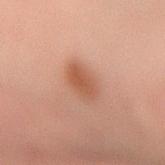Part of a total-body skin-imaging series; this lesion was reviewed on a skin check and was not flagged for biopsy. A close-up tile cropped from a whole-body skin photograph, about 15 mm across. Longest diameter approximately 3 mm. A male patient aged 28–32. The tile uses cross-polarized illumination. The lesion is on the left forearm.